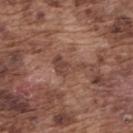Impression:
The lesion was tiled from a total-body skin photograph and was not biopsied.
Background:
The patient is a male aged around 75. The recorded lesion diameter is about 4 mm. Automated image analysis of the tile measured an average lesion color of about L≈43 a*≈20 b*≈25 (CIELAB), a lesion–skin lightness drop of about 8, and a normalized lesion–skin contrast near 6.5. The software also gave a border-irregularity rating of about 7/10 and radial color variation of about 1. It also reported a detector confidence of about 70 out of 100 that the crop contains a lesion. On the back. The tile uses white-light illumination. A close-up tile cropped from a whole-body skin photograph, about 15 mm across.